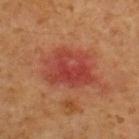| key | value |
|---|---|
| notes | catalogued during a skin exam; not biopsied |
| subject | male, roughly 60 years of age |
| image source | ~15 mm crop, total-body skin-cancer survey |
| lighting | cross-polarized illumination |
| site | the upper back |